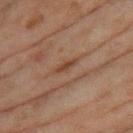notes = no biopsy performed (imaged during a skin exam)
automated lesion analysis = an area of roughly 2 mm², a shape eccentricity near 0.95, and a symmetry-axis asymmetry near 0.3; a nevus-likeness score of about 0/100 and a lesion-detection confidence of about 85/100
subject = female, aged 58–62
lesion size = about 3 mm
tile lighting = cross-polarized illumination
imaging modality = 15 mm crop, total-body photography
body site = the right thigh